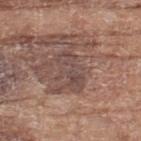notes: no biopsy performed (imaged during a skin exam) | image-analysis metrics: an area of roughly 7.5 mm², a shape eccentricity near 0.9, and a symmetry-axis asymmetry near 0.35; a lesion-to-skin contrast of about 6 (normalized; higher = more distinct); a nevus-likeness score of about 0/100 and a lesion-detection confidence of about 70/100 | patient: female, aged 73–77 | body site: the left thigh | image: ~15 mm tile from a whole-body skin photo.